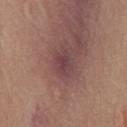Clinical impression:
The lesion was tiled from a total-body skin photograph and was not biopsied.
Background:
A region of skin cropped from a whole-body photographic capture, roughly 15 mm wide. A male patient, aged 53 to 57. Measured at roughly 3.5 mm in maximum diameter. Imaged with white-light lighting. Located on the chest.